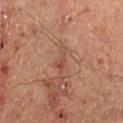follow-up: catalogued during a skin exam; not biopsied | body site: the right lower leg | automated metrics: a footprint of about 3 mm², an outline eccentricity of about 0.85 (0 = round, 1 = elongated), and two-axis asymmetry of about 0.55; roughly 7 lightness units darker than nearby skin and a normalized border contrast of about 5.5; a nevus-likeness score of about 0/100 and a lesion-detection confidence of about 95/100 | patient: male, aged around 50 | tile lighting: cross-polarized illumination | diameter: ≈3 mm | imaging modality: 15 mm crop, total-body photography.